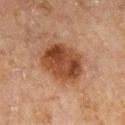Q: Lesion size?
A: ≈5 mm
Q: How was the tile lit?
A: cross-polarized illumination
Q: How was this image acquired?
A: ~15 mm tile from a whole-body skin photo
Q: Where on the body is the lesion?
A: the left lower leg
Q: Patient demographics?
A: male, about 65 years old